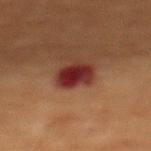<case>
  <biopsy_status>not biopsied; imaged during a skin examination</biopsy_status>
  <patient>
    <sex>female</sex>
    <age_approx>80</age_approx>
  </patient>
  <lesion_size>
    <long_diameter_mm_approx>3.0</long_diameter_mm_approx>
  </lesion_size>
  <lighting>cross-polarized</lighting>
  <automated_metrics>
    <eccentricity>0.4</eccentricity>
    <shape_asymmetry>0.15</shape_asymmetry>
    <nevus_likeness_0_100>0</nevus_likeness_0_100>
    <lesion_detection_confidence_0_100>100</lesion_detection_confidence_0_100>
  </automated_metrics>
  <site>chest</site>
  <image>
    <source>total-body photography crop</source>
    <field_of_view_mm>15</field_of_view_mm>
  </image>
</case>